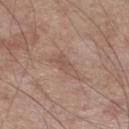Q: Was this lesion biopsied?
A: total-body-photography surveillance lesion; no biopsy
Q: Who is the patient?
A: male, about 65 years old
Q: How large is the lesion?
A: ≈4 mm
Q: Where on the body is the lesion?
A: the leg
Q: Illumination type?
A: white-light
Q: How was this image acquired?
A: 15 mm crop, total-body photography